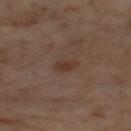This lesion was catalogued during total-body skin photography and was not selected for biopsy. The lesion's longest dimension is about 2.5 mm. A 15 mm close-up tile from a total-body photography series done for melanoma screening. A female patient, about 55 years old. The total-body-photography lesion software estimated a footprint of about 3 mm², an outline eccentricity of about 0.85 (0 = round, 1 = elongated), and two-axis asymmetry of about 0.2. The software also gave a lesion-to-skin contrast of about 6.5 (normalized; higher = more distinct). And it measured an automated nevus-likeness rating near 45 out of 100 and a lesion-detection confidence of about 100/100. From the left thigh. The tile uses cross-polarized illumination.